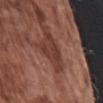Q: Was this lesion biopsied?
A: no biopsy performed (imaged during a skin exam)
Q: Lesion location?
A: the arm
Q: What lighting was used for the tile?
A: white-light illumination
Q: Automated lesion metrics?
A: a footprint of about 8 mm² and a symmetry-axis asymmetry near 0.5; a border-irregularity rating of about 6.5/10, a within-lesion color-variation index near 2.5/10, and radial color variation of about 0.5
Q: What is the imaging modality?
A: 15 mm crop, total-body photography
Q: What is the lesion's diameter?
A: ~4 mm (longest diameter)
Q: Who is the patient?
A: male, approximately 75 years of age